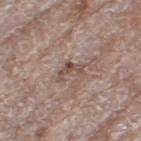Assessment:
The lesion was photographed on a routine skin check and not biopsied; there is no pathology result.
Acquisition and patient details:
On the left thigh. Imaged with white-light lighting. Automated tile analysis of the lesion measured a lesion area of about 3.5 mm² and an outline eccentricity of about 0.8 (0 = round, 1 = elongated). The analysis additionally found a border-irregularity rating of about 8/10, internal color variation of about 0 on a 0–10 scale, and a peripheral color-asymmetry measure near 0. The recorded lesion diameter is about 3 mm. A roughly 15 mm field-of-view crop from a total-body skin photograph. A female patient approximately 75 years of age.